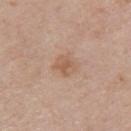No biopsy was performed on this lesion — it was imaged during a full skin examination and was not determined to be concerning. On the left upper arm. The recorded lesion diameter is about 2.5 mm. A female subject aged around 50. Imaged with white-light lighting. A roughly 15 mm field-of-view crop from a total-body skin photograph. The lesion-visualizer software estimated a lesion color around L≈58 a*≈19 b*≈31 in CIELAB, a lesion–skin lightness drop of about 7, and a lesion-to-skin contrast of about 6 (normalized; higher = more distinct).